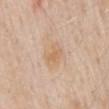This is a white-light tile. A 15 mm close-up tile from a total-body photography series done for melanoma screening. Longest diameter approximately 3.5 mm. On the mid back. A male subject approximately 60 years of age.